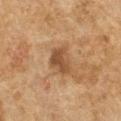biopsy_status: not biopsied; imaged during a skin examination
site: left forearm
automated_metrics:
  nevus_likeness_0_100: 30
image:
  source: total-body photography crop
  field_of_view_mm: 15
lesion_size:
  long_diameter_mm_approx: 3.5
lighting: cross-polarized
patient:
  sex: female
  age_approx: 60